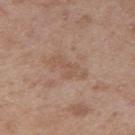Case summary:
– notes: total-body-photography surveillance lesion; no biopsy
– subject: male, aged approximately 50
– body site: the arm
– lesion size: ~4.5 mm (longest diameter)
– TBP lesion metrics: a lesion area of about 6 mm² and a shape-asymmetry score of about 0.4 (0 = symmetric); a lesion color around L≈54 a*≈18 b*≈28 in CIELAB, about 6 CIELAB-L* units darker than the surrounding skin, and a normalized lesion–skin contrast near 4.5; a border-irregularity rating of about 5.5/10 and a within-lesion color-variation index near 1/10; a nevus-likeness score of about 0/100 and a lesion-detection confidence of about 100/100
– imaging modality: 15 mm crop, total-body photography